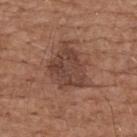biopsy_status: not biopsied; imaged during a skin examination
image:
  source: total-body photography crop
  field_of_view_mm: 15
lighting: white-light
patient:
  sex: male
  age_approx: 75
site: upper back